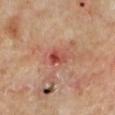notes = no biopsy performed (imaged during a skin exam)
subject = male, in their mid- to late 60s
lighting = cross-polarized illumination
body site = the left lower leg
acquisition = total-body-photography crop, ~15 mm field of view